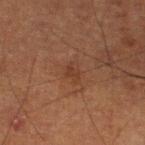notes = catalogued during a skin exam; not biopsied | body site = the leg | illumination = cross-polarized illumination | size = ~2.5 mm (longest diameter) | subject = male, about 75 years old | image = ~15 mm crop, total-body skin-cancer survey | TBP lesion metrics = a border-irregularity rating of about 3/10 and internal color variation of about 1 on a 0–10 scale; a nevus-likeness score of about 0/100.